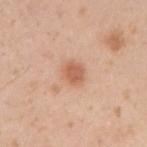• biopsy status · catalogued during a skin exam; not biopsied
• subject · male, aged around 30
• anatomic site · the right upper arm
• image source · 15 mm crop, total-body photography